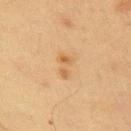biopsy status = no biopsy performed (imaged during a skin exam); anatomic site = the right upper arm; subject = male, approximately 70 years of age; acquisition = total-body-photography crop, ~15 mm field of view.